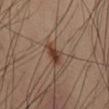Q: Is there a histopathology result?
A: imaged on a skin check; not biopsied
Q: How was this image acquired?
A: ~15 mm crop, total-body skin-cancer survey
Q: What did automated image analysis measure?
A: a footprint of about 3.5 mm², a shape eccentricity near 0.85, and a symmetry-axis asymmetry near 0.3; a border-irregularity rating of about 3/10, a color-variation rating of about 2/10, and peripheral color asymmetry of about 0.5; a lesion-detection confidence of about 100/100
Q: Who is the patient?
A: male, aged 53 to 57
Q: Where on the body is the lesion?
A: the right thigh
Q: How was the tile lit?
A: cross-polarized illumination